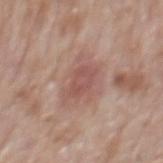Captured during whole-body skin photography for melanoma surveillance; the lesion was not biopsied.
On the back.
This image is a 15 mm lesion crop taken from a total-body photograph.
Automated image analysis of the tile measured an area of roughly 5.5 mm², a shape eccentricity near 0.75, and a shape-asymmetry score of about 0.2 (0 = symmetric). And it measured border irregularity of about 2.5 on a 0–10 scale, internal color variation of about 2.5 on a 0–10 scale, and a peripheral color-asymmetry measure near 0.5.
Measured at roughly 3 mm in maximum diameter.
A male subject, roughly 70 years of age.
This is a white-light tile.